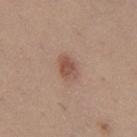Recorded during total-body skin imaging; not selected for excision or biopsy.
From the left thigh.
Measured at roughly 3 mm in maximum diameter.
Cropped from a total-body skin-imaging series; the visible field is about 15 mm.
The total-body-photography lesion software estimated an eccentricity of roughly 0.75 and a shape-asymmetry score of about 0.2 (0 = symmetric).
Imaged with white-light lighting.
The subject is a female aged around 40.